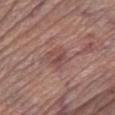Part of a total-body skin-imaging series; this lesion was reviewed on a skin check and was not flagged for biopsy.
Imaged with white-light lighting.
About 3 mm across.
On the left lower leg.
A male subject aged approximately 70.
An algorithmic analysis of the crop reported an average lesion color of about L≈47 a*≈22 b*≈22 (CIELAB), a lesion–skin lightness drop of about 8, and a lesion-to-skin contrast of about 6 (normalized; higher = more distinct). And it measured an automated nevus-likeness rating near 0 out of 100 and lesion-presence confidence of about 100/100.
Cropped from a total-body skin-imaging series; the visible field is about 15 mm.